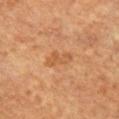follow-up=imaged on a skin check; not biopsied | image=~15 mm tile from a whole-body skin photo | illumination=cross-polarized | site=the chest | lesion size=~3.5 mm (longest diameter) | patient=female, about 60 years old.